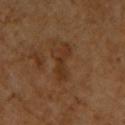Clinical impression:
No biopsy was performed on this lesion — it was imaged during a full skin examination and was not determined to be concerning.
Context:
A male subject aged 58 to 62. Cropped from a whole-body photographic skin survey; the tile spans about 15 mm. Located on the upper back.